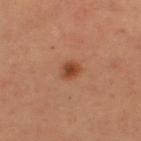Q: Was a biopsy performed?
A: catalogued during a skin exam; not biopsied
Q: Automated lesion metrics?
A: a mean CIELAB color near L≈45 a*≈26 b*≈35 and a lesion–skin lightness drop of about 11; a border-irregularity index near 2/10, a within-lesion color-variation index near 3.5/10, and peripheral color asymmetry of about 1
Q: What are the patient's age and sex?
A: male, approximately 55 years of age
Q: Illumination type?
A: cross-polarized illumination
Q: What is the imaging modality?
A: ~15 mm crop, total-body skin-cancer survey
Q: Lesion size?
A: ~2 mm (longest diameter)
Q: What is the anatomic site?
A: the upper back